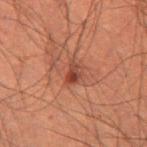Q: Is there a histopathology result?
A: catalogued during a skin exam; not biopsied
Q: What is the lesion's diameter?
A: ≈2.5 mm
Q: What are the patient's age and sex?
A: male, in their 60s
Q: What is the imaging modality?
A: ~15 mm crop, total-body skin-cancer survey
Q: Where on the body is the lesion?
A: the left thigh